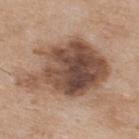Captured during whole-body skin photography for melanoma surveillance; the lesion was not biopsied. A region of skin cropped from a whole-body photographic capture, roughly 15 mm wide. The lesion is on the upper back. The lesion's longest dimension is about 10.5 mm. A male subject aged 73 to 77.